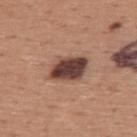Q: Was this lesion biopsied?
A: total-body-photography surveillance lesion; no biopsy
Q: How was this image acquired?
A: 15 mm crop, total-body photography
Q: How was the tile lit?
A: white-light
Q: Who is the patient?
A: male, in their mid-40s
Q: How large is the lesion?
A: about 4.5 mm
Q: Where on the body is the lesion?
A: the upper back